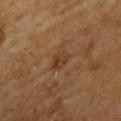Imaged during a routine full-body skin examination; the lesion was not biopsied and no histopathology is available. The total-body-photography lesion software estimated a mean CIELAB color near L≈34 a*≈17 b*≈28 and roughly 6 lightness units darker than nearby skin. And it measured a border-irregularity rating of about 2.5/10 and a color-variation rating of about 3.5/10. The software also gave a nevus-likeness score of about 25/100 and lesion-presence confidence of about 100/100. The lesion is located on the chest. This image is a 15 mm lesion crop taken from a total-body photograph. A male patient, in their mid- to late 60s. The tile uses cross-polarized illumination.